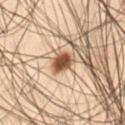Q: Is there a histopathology result?
A: catalogued during a skin exam; not biopsied
Q: Lesion size?
A: ~2.5 mm (longest diameter)
Q: How was the tile lit?
A: cross-polarized illumination
Q: Patient demographics?
A: male, in their 50s
Q: Automated lesion metrics?
A: a normalized border contrast of about 12.5; a border-irregularity rating of about 2/10, a within-lesion color-variation index near 2.5/10, and peripheral color asymmetry of about 1; a nevus-likeness score of about 100/100
Q: What kind of image is this?
A: ~15 mm tile from a whole-body skin photo
Q: What is the anatomic site?
A: the leg